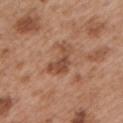Impression: This lesion was catalogued during total-body skin photography and was not selected for biopsy. Acquisition and patient details: A male patient aged approximately 55. This image is a 15 mm lesion crop taken from a total-body photograph. On the left upper arm. Automated image analysis of the tile measured a lesion color around L≈48 a*≈22 b*≈31 in CIELAB, roughly 10 lightness units darker than nearby skin, and a lesion-to-skin contrast of about 7.5 (normalized; higher = more distinct). Longest diameter approximately 4 mm. Imaged with white-light lighting.